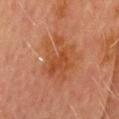<tbp_lesion>
<biopsy_status>not biopsied; imaged during a skin examination</biopsy_status>
<image>
  <source>total-body photography crop</source>
  <field_of_view_mm>15</field_of_view_mm>
</image>
<patient>
  <sex>male</sex>
  <age_approx>70</age_approx>
</patient>
<site>head or neck</site>
</tbp_lesion>